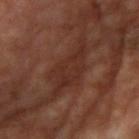Part of a total-body skin-imaging series; this lesion was reviewed on a skin check and was not flagged for biopsy. A male subject roughly 65 years of age. A 15 mm close-up extracted from a 3D total-body photography capture. The lesion is located on the right upper arm.